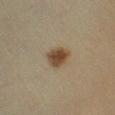No biopsy was performed on this lesion — it was imaged during a full skin examination and was not determined to be concerning. Measured at roughly 3 mm in maximum diameter. A female subject aged approximately 40. On the leg. A region of skin cropped from a whole-body photographic capture, roughly 15 mm wide.